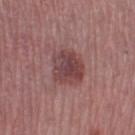The lesion was photographed on a routine skin check and not biopsied; there is no pathology result.
The tile uses white-light illumination.
A female patient approximately 45 years of age.
A 15 mm close-up tile from a total-body photography series done for melanoma screening.
On the left thigh.